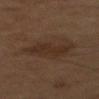follow-up: imaged on a skin check; not biopsied
diameter: ~4.5 mm (longest diameter)
patient: male, roughly 60 years of age
automated lesion analysis: a lesion color around L≈25 a*≈13 b*≈22 in CIELAB and roughly 5 lightness units darker than nearby skin
body site: the chest
image: ~15 mm tile from a whole-body skin photo
lighting: cross-polarized illumination